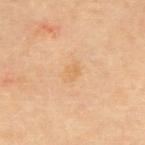Clinical impression: Part of a total-body skin-imaging series; this lesion was reviewed on a skin check and was not flagged for biopsy.